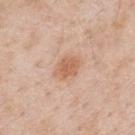workup — imaged on a skin check; not biopsied | body site — the mid back | image — total-body-photography crop, ~15 mm field of view | patient — male, aged 48–52.